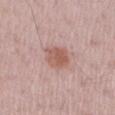Clinical impression:
This lesion was catalogued during total-body skin photography and was not selected for biopsy.
Background:
A close-up tile cropped from a whole-body skin photograph, about 15 mm across. On the right upper arm. A female patient aged approximately 45. Automated tile analysis of the lesion measured a lesion color around L≈57 a*≈22 b*≈26 in CIELAB, a lesion–skin lightness drop of about 10, and a normalized lesion–skin contrast near 7.5. The software also gave a classifier nevus-likeness of about 75/100 and a detector confidence of about 100 out of 100 that the crop contains a lesion. This is a white-light tile. Measured at roughly 3 mm in maximum diameter.